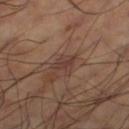Impression:
Recorded during total-body skin imaging; not selected for excision or biopsy.
Acquisition and patient details:
About 2.5 mm across. On the left thigh. A male subject, roughly 60 years of age. A lesion tile, about 15 mm wide, cut from a 3D total-body photograph. Imaged with cross-polarized lighting.